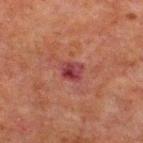This lesion was catalogued during total-body skin photography and was not selected for biopsy. Cropped from a whole-body photographic skin survey; the tile spans about 15 mm. Approximately 2.5 mm at its widest. From the upper back. The patient is a male about 60 years old. This is a cross-polarized tile.